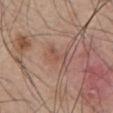Assessment: Part of a total-body skin-imaging series; this lesion was reviewed on a skin check and was not flagged for biopsy. Clinical summary: This is a white-light tile. A 15 mm crop from a total-body photograph taken for skin-cancer surveillance. The patient is a male approximately 55 years of age. On the upper back.